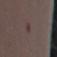Q: Is there a histopathology result?
A: imaged on a skin check; not biopsied
Q: How large is the lesion?
A: about 3 mm
Q: What is the imaging modality?
A: 15 mm crop, total-body photography
Q: Patient demographics?
A: female, approximately 30 years of age
Q: How was the tile lit?
A: white-light
Q: Where on the body is the lesion?
A: the right upper arm
Q: Automated lesion metrics?
A: a mean CIELAB color near L≈37 a*≈16 b*≈15, roughly 5 lightness units darker than nearby skin, and a normalized border contrast of about 4.5